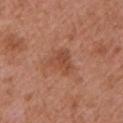– notes: imaged on a skin check; not biopsied
– image source: total-body-photography crop, ~15 mm field of view
– illumination: white-light illumination
– TBP lesion metrics: a border-irregularity rating of about 4/10
– location: the left upper arm
– subject: male, about 75 years old
– size: ≈3.5 mm